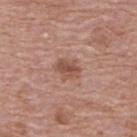A female subject, aged around 65.
From the upper back.
Approximately 3 mm at its widest.
This image is a 15 mm lesion crop taken from a total-body photograph.